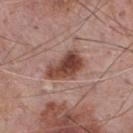Recorded during total-body skin imaging; not selected for excision or biopsy. The lesion's longest dimension is about 5 mm. A roughly 15 mm field-of-view crop from a total-body skin photograph. From the chest. The lesion-visualizer software estimated a mean CIELAB color near L≈45 a*≈22 b*≈26 and a lesion-to-skin contrast of about 10.5 (normalized; higher = more distinct). A male patient, about 75 years old. Imaged with white-light lighting.